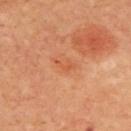| field | value |
|---|---|
| subject | male, aged around 65 |
| body site | the upper back |
| image | ~15 mm tile from a whole-body skin photo |
| illumination | cross-polarized illumination |